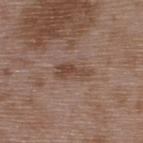Impression:
This lesion was catalogued during total-body skin photography and was not selected for biopsy.
Clinical summary:
Approximately 4 mm at its widest. An algorithmic analysis of the crop reported a lesion area of about 4.5 mm². It also reported a lesion color around L≈44 a*≈18 b*≈25 in CIELAB, roughly 9 lightness units darker than nearby skin, and a normalized border contrast of about 7. It also reported lesion-presence confidence of about 100/100. The tile uses white-light illumination. This image is a 15 mm lesion crop taken from a total-body photograph. The lesion is located on the upper back. The patient is a male aged around 50.